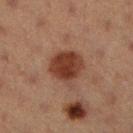| field | value |
|---|---|
| biopsy status | catalogued during a skin exam; not biopsied |
| subject | female, approximately 40 years of age |
| imaging modality | ~15 mm tile from a whole-body skin photo |
| body site | the right lower leg |